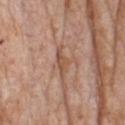No biopsy was performed on this lesion — it was imaged during a full skin examination and was not determined to be concerning. The recorded lesion diameter is about 4.5 mm. A 15 mm close-up tile from a total-body photography series done for melanoma screening. A female patient roughly 70 years of age. Located on the chest.